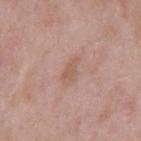follow-up = imaged on a skin check; not biopsied
subject = male, roughly 55 years of age
site = the mid back
imaging modality = ~15 mm tile from a whole-body skin photo
illumination = white-light
image-analysis metrics = a mean CIELAB color near L≈57 a*≈20 b*≈28, roughly 7 lightness units darker than nearby skin, and a normalized border contrast of about 6
diameter = ≈3 mm